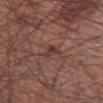Assessment:
Captured during whole-body skin photography for melanoma surveillance; the lesion was not biopsied.
Image and clinical context:
A roughly 15 mm field-of-view crop from a total-body skin photograph. The recorded lesion diameter is about 2.5 mm. The tile uses white-light illumination. The subject is a male in their mid- to late 60s. Located on the right upper arm. Automated tile analysis of the lesion measured a lesion area of about 3 mm² and an eccentricity of roughly 0.6. The software also gave roughly 8 lightness units darker than nearby skin and a normalized lesion–skin contrast near 6.5. The analysis additionally found border irregularity of about 4 on a 0–10 scale and a within-lesion color-variation index near 3.5/10.